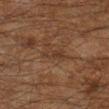follow-up = total-body-photography surveillance lesion; no biopsy
anatomic site = the right lower leg
patient = male, aged 58 to 62
lighting = cross-polarized
automated lesion analysis = a footprint of about 3 mm² and two-axis asymmetry of about 0.3; a border-irregularity rating of about 3/10, a color-variation rating of about 2/10, and peripheral color asymmetry of about 0.5; a nevus-likeness score of about 0/100 and a lesion-detection confidence of about 60/100
imaging modality = ~15 mm tile from a whole-body skin photo